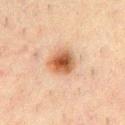Findings:
• follow-up · imaged on a skin check; not biopsied
• tile lighting · cross-polarized
• patient · male, roughly 50 years of age
• lesion size · about 4 mm
• image source · ~15 mm crop, total-body skin-cancer survey
• automated metrics · a lesion area of about 10 mm², an eccentricity of roughly 0.45, and two-axis asymmetry of about 0.15; a classifier nevus-likeness of about 95/100
• anatomic site · the chest